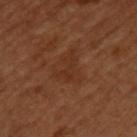Case summary:
- follow-up — total-body-photography surveillance lesion; no biopsy
- automated lesion analysis — a footprint of about 10 mm² and an outline eccentricity of about 0.65 (0 = round, 1 = elongated); an average lesion color of about L≈32 a*≈21 b*≈30 (CIELAB) and a normalized lesion–skin contrast near 5; border irregularity of about 4.5 on a 0–10 scale and a within-lesion color-variation index near 2.5/10; a classifier nevus-likeness of about 0/100 and a detector confidence of about 100 out of 100 that the crop contains a lesion
- patient — male, approximately 50 years of age
- illumination — cross-polarized
- site — the upper back
- lesion size — ≈4.5 mm
- image source — ~15 mm crop, total-body skin-cancer survey